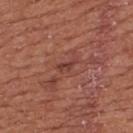No biopsy was performed on this lesion — it was imaged during a full skin examination and was not determined to be concerning.
The subject is a male in their mid-60s.
The tile uses white-light illumination.
Located on the upper back.
The lesion-visualizer software estimated an average lesion color of about L≈40 a*≈25 b*≈25 (CIELAB), roughly 8 lightness units darker than nearby skin, and a lesion-to-skin contrast of about 7 (normalized; higher = more distinct). The analysis additionally found border irregularity of about 4.5 on a 0–10 scale, a within-lesion color-variation index near 0/10, and a peripheral color-asymmetry measure near 0. The analysis additionally found an automated nevus-likeness rating near 0 out of 100 and lesion-presence confidence of about 80/100.
A roughly 15 mm field-of-view crop from a total-body skin photograph.